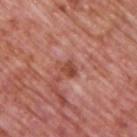Background: An algorithmic analysis of the crop reported about 10 CIELAB-L* units darker than the surrounding skin. A male subject, aged 73 to 77. Measured at roughly 2.5 mm in maximum diameter. Captured under white-light illumination. A lesion tile, about 15 mm wide, cut from a 3D total-body photograph. Located on the upper back.